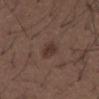Q: Was this lesion biopsied?
A: total-body-photography surveillance lesion; no biopsy
Q: What are the patient's age and sex?
A: male, aged 48 to 52
Q: Lesion size?
A: ~3 mm (longest diameter)
Q: What is the imaging modality?
A: 15 mm crop, total-body photography
Q: Automated lesion metrics?
A: a footprint of about 4.5 mm², an outline eccentricity of about 0.75 (0 = round, 1 = elongated), and a symmetry-axis asymmetry near 0.2; a lesion–skin lightness drop of about 7 and a normalized border contrast of about 7; an automated nevus-likeness rating near 55 out of 100
Q: How was the tile lit?
A: white-light illumination
Q: What is the anatomic site?
A: the mid back